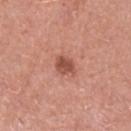follow-up: total-body-photography surveillance lesion; no biopsy | patient: male, aged 48–52 | size: ≈2.5 mm | tile lighting: white-light | acquisition: ~15 mm tile from a whole-body skin photo | body site: the left lower leg.